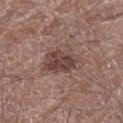| key | value |
|---|---|
| follow-up | catalogued during a skin exam; not biopsied |
| anatomic site | the leg |
| patient | male, aged around 70 |
| tile lighting | white-light |
| TBP lesion metrics | a footprint of about 11 mm², an outline eccentricity of about 0.75 (0 = round, 1 = elongated), and a symmetry-axis asymmetry near 0.2; border irregularity of about 2.5 on a 0–10 scale and a peripheral color-asymmetry measure near 1.5 |
| lesion size | ≈4.5 mm |
| image source | total-body-photography crop, ~15 mm field of view |